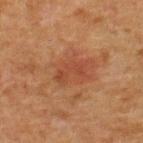biopsy status=no biopsy performed (imaged during a skin exam); location=the upper back; imaging modality=~15 mm crop, total-body skin-cancer survey; subject=male, aged approximately 50.